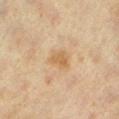Part of a total-body skin-imaging series; this lesion was reviewed on a skin check and was not flagged for biopsy. Located on the left lower leg. The patient is a female aged 38–42. Measured at roughly 3 mm in maximum diameter. This is a cross-polarized tile. The total-body-photography lesion software estimated a footprint of about 4.5 mm², a shape eccentricity near 0.7, and a shape-asymmetry score of about 0.4 (0 = symmetric). A roughly 15 mm field-of-view crop from a total-body skin photograph.